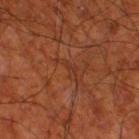biopsy status: imaged on a skin check; not biopsied
site: the right thigh
subject: male, aged around 70
acquisition: total-body-photography crop, ~15 mm field of view